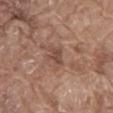From the mid back. Automated image analysis of the tile measured a footprint of about 3.5 mm², an outline eccentricity of about 0.7 (0 = round, 1 = elongated), and two-axis asymmetry of about 0.35. And it measured a mean CIELAB color near L≈46 a*≈20 b*≈25, roughly 8 lightness units darker than nearby skin, and a normalized lesion–skin contrast near 6.5. The analysis additionally found a border-irregularity index near 3.5/10, internal color variation of about 1 on a 0–10 scale, and radial color variation of about 0.5. The lesion's longest dimension is about 2.5 mm. The tile uses white-light illumination. A 15 mm crop from a total-body photograph taken for skin-cancer surveillance. A male subject, aged around 80.